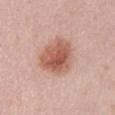Case summary:
- workup: total-body-photography surveillance lesion; no biopsy
- illumination: white-light illumination
- image-analysis metrics: a border-irregularity rating of about 2/10, a within-lesion color-variation index near 6/10, and a peripheral color-asymmetry measure near 2; a nevus-likeness score of about 100/100 and a detector confidence of about 100 out of 100 that the crop contains a lesion
- patient: male, approximately 40 years of age
- image: ~15 mm tile from a whole-body skin photo
- lesion size: ~5 mm (longest diameter)
- body site: the abdomen